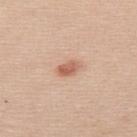workup: catalogued during a skin exam; not biopsied | acquisition: ~15 mm crop, total-body skin-cancer survey | subject: female, in their mid-50s | body site: the upper back | lesion diameter: ~2.5 mm (longest diameter) | tile lighting: white-light illumination | TBP lesion metrics: a footprint of about 3.5 mm², an eccentricity of roughly 0.8, and a shape-asymmetry score of about 0.25 (0 = symmetric); an average lesion color of about L≈61 a*≈24 b*≈31 (CIELAB), about 12 CIELAB-L* units darker than the surrounding skin, and a lesion-to-skin contrast of about 7.5 (normalized; higher = more distinct).